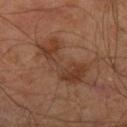On the right leg. A 15 mm crop from a total-body photograph taken for skin-cancer surveillance. The total-body-photography lesion software estimated a footprint of about 16 mm², an eccentricity of roughly 0.9, and a symmetry-axis asymmetry near 0.5. The software also gave border irregularity of about 7.5 on a 0–10 scale and internal color variation of about 4 on a 0–10 scale. The subject is a male aged 58–62. Measured at roughly 7 mm in maximum diameter. Imaged with cross-polarized lighting.